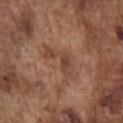| field | value |
|---|---|
| workup | catalogued during a skin exam; not biopsied |
| lesion diameter | ~5.5 mm (longest diameter) |
| image source | total-body-photography crop, ~15 mm field of view |
| patient | male, roughly 75 years of age |
| illumination | white-light illumination |
| anatomic site | the chest |
| image-analysis metrics | an area of roughly 8 mm², an eccentricity of roughly 0.9, and a shape-asymmetry score of about 0.65 (0 = symmetric); a mean CIELAB color near L≈44 a*≈21 b*≈29, roughly 7 lightness units darker than nearby skin, and a lesion-to-skin contrast of about 6 (normalized; higher = more distinct); a color-variation rating of about 2.5/10 |